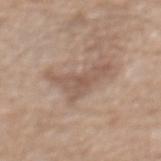follow-up = no biopsy performed (imaged during a skin exam) | illumination = white-light illumination | location = the front of the torso | subject = male, aged 68 to 72 | image source = ~15 mm crop, total-body skin-cancer survey | lesion diameter = ~5.5 mm (longest diameter).